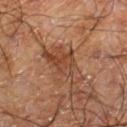biopsy status = imaged on a skin check; not biopsied | site = the right lower leg | patient = male, aged approximately 60 | image source = ~15 mm crop, total-body skin-cancer survey.